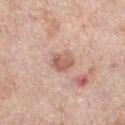Case summary:
– notes: imaged on a skin check; not biopsied
– illumination: white-light
– location: the leg
– image source: total-body-photography crop, ~15 mm field of view
– patient: female, aged 63–67
– diameter: about 3 mm